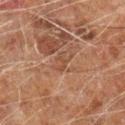Background: The subject is a male aged approximately 60. The total-body-photography lesion software estimated an area of roughly 2 mm², a shape eccentricity near 0.9, and a symmetry-axis asymmetry near 0.7. And it measured a border-irregularity index near 7.5/10, a within-lesion color-variation index near 0/10, and radial color variation of about 0. It also reported a nevus-likeness score of about 0/100 and a lesion-detection confidence of about 60/100. The recorded lesion diameter is about 2.5 mm. This image is a 15 mm lesion crop taken from a total-body photograph. Located on the left forearm.